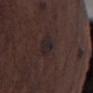| key | value |
|---|---|
| notes | total-body-photography surveillance lesion; no biopsy |
| patient | male, about 75 years old |
| imaging modality | total-body-photography crop, ~15 mm field of view |
| illumination | white-light |
| site | the left thigh |
| diameter | ≈3 mm |